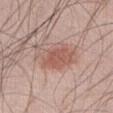Background: The subject is a male aged approximately 60. A 15 mm close-up extracted from a 3D total-body photography capture. On the front of the torso. Imaged with white-light lighting. The recorded lesion diameter is about 6 mm.